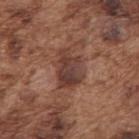notes: catalogued during a skin exam; not biopsied | imaging modality: ~15 mm tile from a whole-body skin photo | patient: male, aged around 75 | lesion diameter: ≈5 mm | automated metrics: a footprint of about 12 mm², an eccentricity of roughly 0.75, and a shape-asymmetry score of about 0.25 (0 = symmetric); a border-irregularity rating of about 3/10, internal color variation of about 5 on a 0–10 scale, and peripheral color asymmetry of about 1.5; a nevus-likeness score of about 0/100 and lesion-presence confidence of about 100/100 | site: the arm | lighting: white-light.